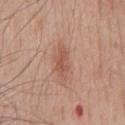Impression:
Captured during whole-body skin photography for melanoma surveillance; the lesion was not biopsied.
Image and clinical context:
On the chest. Automated tile analysis of the lesion measured a lesion–skin lightness drop of about 9. And it measured border irregularity of about 3.5 on a 0–10 scale, a color-variation rating of about 2.5/10, and a peripheral color-asymmetry measure near 1. And it measured a nevus-likeness score of about 70/100 and a detector confidence of about 100 out of 100 that the crop contains a lesion. A male subject, in their 60s. Approximately 4 mm at its widest. A 15 mm close-up extracted from a 3D total-body photography capture.